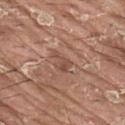Impression: Part of a total-body skin-imaging series; this lesion was reviewed on a skin check and was not flagged for biopsy.